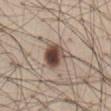  biopsy_status: not biopsied; imaged during a skin examination
  lesion_size:
    long_diameter_mm_approx: 4.0
  image:
    source: total-body photography crop
    field_of_view_mm: 15
  site: abdomen
  lighting: white-light
  patient:
    sex: male
    age_approx: 60
  automated_metrics:
    border_irregularity_0_10: 3.0
    peripheral_color_asymmetry: 4.0
    nevus_likeness_0_100: 95
    lesion_detection_confidence_0_100: 100